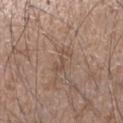Assessment: The lesion was photographed on a routine skin check and not biopsied; there is no pathology result. Image and clinical context: The tile uses white-light illumination. Measured at roughly 3.5 mm in maximum diameter. A region of skin cropped from a whole-body photographic capture, roughly 15 mm wide. A male patient roughly 50 years of age. The lesion is located on the right forearm.